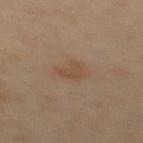This lesion was catalogued during total-body skin photography and was not selected for biopsy. On the upper back. This is a cross-polarized tile. A 15 mm close-up tile from a total-body photography series done for melanoma screening. Approximately 2.5 mm at its widest. A female subject, about 40 years old.